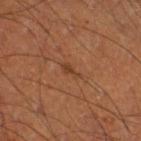The lesion was tiled from a total-body skin photograph and was not biopsied.
Located on the leg.
A close-up tile cropped from a whole-body skin photograph, about 15 mm across.
The lesion's longest dimension is about 2.5 mm.
A male patient, aged 48 to 52.